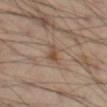| field | value |
|---|---|
| biopsy status | imaged on a skin check; not biopsied |
| illumination | cross-polarized |
| lesion diameter | ≈2.5 mm |
| body site | the left thigh |
| imaging modality | 15 mm crop, total-body photography |
| patient | male, roughly 55 years of age |
| automated lesion analysis | a footprint of about 4 mm² |